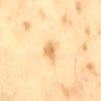Q: Was this lesion biopsied?
A: no biopsy performed (imaged during a skin exam)
Q: Who is the patient?
A: male, in their mid-60s
Q: Automated lesion metrics?
A: an average lesion color of about L≈74 a*≈19 b*≈45 (CIELAB) and roughly 12 lightness units darker than nearby skin; a border-irregularity index near 2.5/10, internal color variation of about 2.5 on a 0–10 scale, and peripheral color asymmetry of about 0.5; an automated nevus-likeness rating near 80 out of 100 and a detector confidence of about 100 out of 100 that the crop contains a lesion
Q: How was this image acquired?
A: ~15 mm tile from a whole-body skin photo